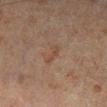This lesion was catalogued during total-body skin photography and was not selected for biopsy.
A close-up tile cropped from a whole-body skin photograph, about 15 mm across.
A male patient aged approximately 70.
This is a cross-polarized tile.
The lesion is located on the right lower leg.
Automated image analysis of the tile measured two-axis asymmetry of about 0.45. The analysis additionally found internal color variation of about 0 on a 0–10 scale and a peripheral color-asymmetry measure near 0. And it measured a detector confidence of about 100 out of 100 that the crop contains a lesion.